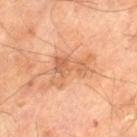This lesion was catalogued during total-body skin photography and was not selected for biopsy. The lesion's longest dimension is about 5.5 mm. The patient is a male about 70 years old. From the left thigh. Automated image analysis of the tile measured a footprint of about 10 mm². It also reported a nevus-likeness score of about 0/100 and a detector confidence of about 100 out of 100 that the crop contains a lesion. Imaged with cross-polarized lighting. A 15 mm crop from a total-body photograph taken for skin-cancer surveillance.